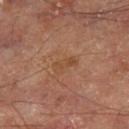This lesion was catalogued during total-body skin photography and was not selected for biopsy. A male patient roughly 70 years of age. The lesion is located on the right thigh. A 15 mm crop from a total-body photograph taken for skin-cancer surveillance. Longest diameter approximately 3 mm.